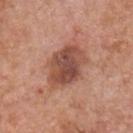The lesion was photographed on a routine skin check and not biopsied; there is no pathology result.
About 5.5 mm across.
Captured under white-light illumination.
From the chest.
The total-body-photography lesion software estimated a lesion area of about 17 mm² and an eccentricity of roughly 0.75. It also reported a border-irregularity rating of about 2/10 and a peripheral color-asymmetry measure near 1.5. It also reported a classifier nevus-likeness of about 50/100 and lesion-presence confidence of about 100/100.
A 15 mm close-up extracted from a 3D total-body photography capture.
A male subject, about 60 years old.